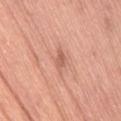<case>
  <biopsy_status>not biopsied; imaged during a skin examination</biopsy_status>
  <lesion_size>
    <long_diameter_mm_approx>2.5</long_diameter_mm_approx>
  </lesion_size>
  <site>leg</site>
  <image>
    <source>total-body photography crop</source>
    <field_of_view_mm>15</field_of_view_mm>
  </image>
  <automated_metrics>
    <vs_skin_darker_L>9.0</vs_skin_darker_L>
    <vs_skin_contrast_norm>6.0</vs_skin_contrast_norm>
    <border_irregularity_0_10>3.5</border_irregularity_0_10>
    <color_variation_0_10>1.5</color_variation_0_10>
    <peripheral_color_asymmetry>0.5</peripheral_color_asymmetry>
    <lesion_detection_confidence_0_100>100</lesion_detection_confidence_0_100>
  </automated_metrics>
  <lighting>white-light</lighting>
  <patient>
    <sex>female</sex>
    <age_approx>55</age_approx>
  </patient>
</case>